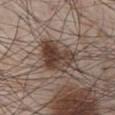{
  "biopsy_status": "not biopsied; imaged during a skin examination",
  "lesion_size": {
    "long_diameter_mm_approx": 5.5
  },
  "lighting": "white-light",
  "site": "chest",
  "automated_metrics": {
    "eccentricity": 0.7,
    "shape_asymmetry": 0.4,
    "cielab_L": 41,
    "cielab_a": 15,
    "cielab_b": 23,
    "vs_skin_darker_L": 12.0,
    "vs_skin_contrast_norm": 10.0,
    "border_irregularity_0_10": 4.5,
    "color_variation_0_10": 7.0,
    "peripheral_color_asymmetry": 2.5
  },
  "patient": {
    "sex": "male",
    "age_approx": 65
  },
  "image": {
    "source": "total-body photography crop",
    "field_of_view_mm": 15
  }
}